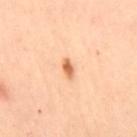Impression: Recorded during total-body skin imaging; not selected for excision or biopsy. Acquisition and patient details: This is a cross-polarized tile. A 15 mm close-up tile from a total-body photography series done for melanoma screening. The lesion-visualizer software estimated a footprint of about 2.5 mm², an outline eccentricity of about 0.85 (0 = round, 1 = elongated), and a shape-asymmetry score of about 0.35 (0 = symmetric). The analysis additionally found a mean CIELAB color near L≈69 a*≈27 b*≈42, roughly 15 lightness units darker than nearby skin, and a normalized border contrast of about 9. It also reported radial color variation of about 0.5. The lesion is on the left leg. A female subject aged 38 to 42.